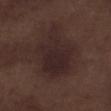Recorded during total-body skin imaging; not selected for excision or biopsy.